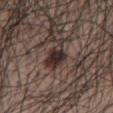Notes:
* notes · imaged on a skin check; not biopsied
* lesion diameter · ≈4.5 mm
* subject · male, aged 68–72
* anatomic site · the mid back
* TBP lesion metrics · a lesion color around L≈30 a*≈14 b*≈17 in CIELAB and a normalized lesion–skin contrast near 11; lesion-presence confidence of about 85/100
* illumination · white-light
* image source · 15 mm crop, total-body photography